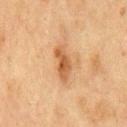Clinical impression:
Recorded during total-body skin imaging; not selected for excision or biopsy.
Acquisition and patient details:
Longest diameter approximately 4 mm. Located on the chest. Captured under cross-polarized illumination. A 15 mm close-up extracted from a 3D total-body photography capture. Automated tile analysis of the lesion measured a lesion area of about 6 mm², a shape eccentricity near 0.9, and a symmetry-axis asymmetry near 0.35. A male patient approximately 75 years of age.